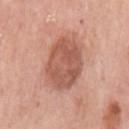This lesion was catalogued during total-body skin photography and was not selected for biopsy.
This image is a 15 mm lesion crop taken from a total-body photograph.
A female subject, in their mid- to late 60s.
Measured at roughly 5.5 mm in maximum diameter.
Imaged with white-light lighting.
On the left upper arm.